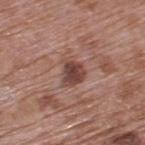notes = total-body-photography surveillance lesion; no biopsy
lesion diameter = ≈3 mm
image = total-body-photography crop, ~15 mm field of view
illumination = white-light illumination
patient = male, approximately 70 years of age
site = the upper back